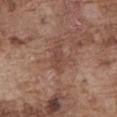Q: Is there a histopathology result?
A: no biopsy performed (imaged during a skin exam)
Q: Where on the body is the lesion?
A: the abdomen
Q: What is the imaging modality?
A: ~15 mm crop, total-body skin-cancer survey
Q: Automated lesion metrics?
A: an average lesion color of about L≈45 a*≈20 b*≈26 (CIELAB) and about 7 CIELAB-L* units darker than the surrounding skin; a classifier nevus-likeness of about 0/100 and lesion-presence confidence of about 100/100
Q: What are the patient's age and sex?
A: male, approximately 75 years of age